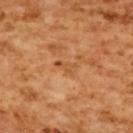Q: Was this lesion biopsied?
A: catalogued during a skin exam; not biopsied
Q: What is the imaging modality?
A: ~15 mm crop, total-body skin-cancer survey
Q: What is the anatomic site?
A: the upper back
Q: How was the tile lit?
A: cross-polarized illumination
Q: Who is the patient?
A: female, in their mid-50s
Q: What is the lesion's diameter?
A: ≈2.5 mm
Q: Automated lesion metrics?
A: a mean CIELAB color near L≈54 a*≈26 b*≈43, roughly 8 lightness units darker than nearby skin, and a lesion-to-skin contrast of about 5.5 (normalized; higher = more distinct); a border-irregularity rating of about 3.5/10, a color-variation rating of about 0/10, and a peripheral color-asymmetry measure near 0